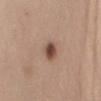Imaged during a routine full-body skin examination; the lesion was not biopsied and no histopathology is available.